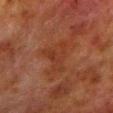The lesion was photographed on a routine skin check and not biopsied; there is no pathology result. Imaged with cross-polarized lighting. A male patient roughly 80 years of age. A close-up tile cropped from a whole-body skin photograph, about 15 mm across. The lesion is located on the left lower leg. Approximately 4.5 mm at its widest.